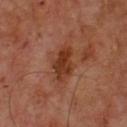Assessment:
No biopsy was performed on this lesion — it was imaged during a full skin examination and was not determined to be concerning.
Acquisition and patient details:
A male patient, in their mid-60s. A 15 mm close-up extracted from a 3D total-body photography capture. Imaged with cross-polarized lighting. The lesion's longest dimension is about 4 mm.